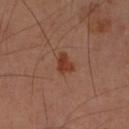This lesion was catalogued during total-body skin photography and was not selected for biopsy. Cropped from a total-body skin-imaging series; the visible field is about 15 mm. On the left forearm. A male patient, in their mid-60s. Captured under cross-polarized illumination. The lesion's longest dimension is about 3 mm.